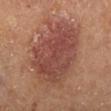The lesion was tiled from a total-body skin photograph and was not biopsied. Located on the right lower leg. Cropped from a total-body skin-imaging series; the visible field is about 15 mm. Imaged with cross-polarized lighting. The recorded lesion diameter is about 9 mm. The patient is a male in their mid-60s. The lesion-visualizer software estimated a classifier nevus-likeness of about 90/100 and a lesion-detection confidence of about 100/100.